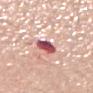<case>
  <biopsy_status>not biopsied; imaged during a skin examination</biopsy_status>
  <image>
    <source>total-body photography crop</source>
    <field_of_view_mm>15</field_of_view_mm>
  </image>
  <site>back</site>
  <lesion_size>
    <long_diameter_mm_approx>2.5</long_diameter_mm_approx>
  </lesion_size>
  <lighting>white-light</lighting>
  <patient>
    <sex>male</sex>
    <age_approx>70</age_approx>
  </patient>
</case>